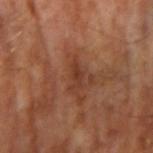• workup: imaged on a skin check; not biopsied
• patient: male, roughly 65 years of age
• image source: ~15 mm crop, total-body skin-cancer survey
• tile lighting: cross-polarized illumination
• lesion size: ≈3 mm
• body site: the right upper arm